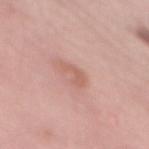The lesion was tiled from a total-body skin photograph and was not biopsied.
Longest diameter approximately 4 mm.
This is a white-light tile.
A 15 mm crop from a total-body photograph taken for skin-cancer surveillance.
Automated tile analysis of the lesion measured an area of roughly 3.5 mm², an outline eccentricity of about 0.95 (0 = round, 1 = elongated), and a shape-asymmetry score of about 0.45 (0 = symmetric). And it measured a mean CIELAB color near L≈60 a*≈23 b*≈28, about 8 CIELAB-L* units darker than the surrounding skin, and a normalized lesion–skin contrast near 6. And it measured internal color variation of about 0 on a 0–10 scale and peripheral color asymmetry of about 0. The software also gave a nevus-likeness score of about 0/100.
The lesion is on the back.
The subject is a female aged 58 to 62.